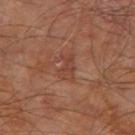Captured during whole-body skin photography for melanoma surveillance; the lesion was not biopsied.
Cropped from a whole-body photographic skin survey; the tile spans about 15 mm.
This is a cross-polarized tile.
The lesion-visualizer software estimated a footprint of about 4.5 mm², an eccentricity of roughly 0.85, and a symmetry-axis asymmetry near 0.3.
A male patient roughly 60 years of age.
Longest diameter approximately 3 mm.
The lesion is on the right leg.